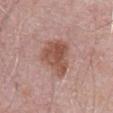Notes:
* imaging modality — ~15 mm crop, total-body skin-cancer survey
* TBP lesion metrics — a lesion area of about 14 mm² and an outline eccentricity of about 0.7 (0 = round, 1 = elongated); a border-irregularity index near 4/10, a color-variation rating of about 4/10, and peripheral color asymmetry of about 1.5; a nevus-likeness score of about 45/100 and a lesion-detection confidence of about 100/100
* diameter — about 5 mm
* body site — the abdomen
* patient — male, aged 58 to 62
* lighting — white-light